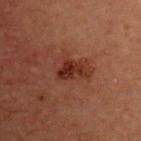<lesion>
  <biopsy_status>not biopsied; imaged during a skin examination</biopsy_status>
  <lighting>cross-polarized</lighting>
  <lesion_size>
    <long_diameter_mm_approx>3.5</long_diameter_mm_approx>
  </lesion_size>
  <automated_metrics>
    <cielab_L>22</cielab_L>
    <cielab_a>21</cielab_a>
    <cielab_b>23</cielab_b>
    <vs_skin_darker_L>9.0</vs_skin_darker_L>
    <vs_skin_contrast_norm>9.5</vs_skin_contrast_norm>
    <border_irregularity_0_10>4.5</border_irregularity_0_10>
    <color_variation_0_10>2.5</color_variation_0_10>
    <peripheral_color_asymmetry>1.0</peripheral_color_asymmetry>
  </automated_metrics>
  <site>front of the torso</site>
  <image>
    <source>total-body photography crop</source>
    <field_of_view_mm>15</field_of_view_mm>
  </image>
  <patient>
    <sex>male</sex>
    <age_approx>60</age_approx>
  </patient>
</lesion>